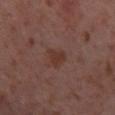Assessment: Imaged during a routine full-body skin examination; the lesion was not biopsied and no histopathology is available. Acquisition and patient details: The lesion is located on the right thigh. About 2.5 mm across. Automated tile analysis of the lesion measured a lesion area of about 4 mm². This is a cross-polarized tile. A region of skin cropped from a whole-body photographic capture, roughly 15 mm wide. A female subject, roughly 55 years of age.